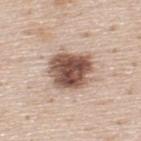Located on the upper back. A close-up tile cropped from a whole-body skin photograph, about 15 mm across. About 5 mm across. This is a white-light tile. A male subject in their mid-40s.